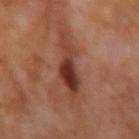Notes:
– biopsy status · no biopsy performed (imaged during a skin exam)
– imaging modality · ~15 mm crop, total-body skin-cancer survey
– anatomic site · the right forearm
– tile lighting · cross-polarized illumination
– patient · female, aged 48 to 52